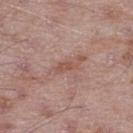anatomic site: the right thigh | image-analysis metrics: a footprint of about 3 mm², an outline eccentricity of about 0.9 (0 = round, 1 = elongated), and a symmetry-axis asymmetry near 0.3; a peripheral color-asymmetry measure near 0; a classifier nevus-likeness of about 0/100 and a detector confidence of about 100 out of 100 that the crop contains a lesion | illumination: white-light illumination | subject: male, aged 48 to 52 | size: about 3 mm | acquisition: total-body-photography crop, ~15 mm field of view.